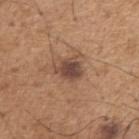body site: the right upper arm
illumination: white-light illumination
size: ≈3.5 mm
patient: male, roughly 65 years of age
image: 15 mm crop, total-body photography